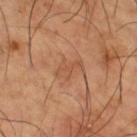<record>
<biopsy_status>not biopsied; imaged during a skin examination</biopsy_status>
<image>
  <source>total-body photography crop</source>
  <field_of_view_mm>15</field_of_view_mm>
</image>
<site>leg</site>
<patient>
  <sex>male</sex>
  <age_approx>65</age_approx>
</patient>
<lesion_size>
  <long_diameter_mm_approx>3.5</long_diameter_mm_approx>
</lesion_size>
<lighting>cross-polarized</lighting>
</record>